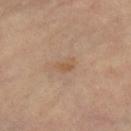{"biopsy_status": "not biopsied; imaged during a skin examination", "patient": {"sex": "female", "age_approx": 65}, "automated_metrics": {"area_mm2_approx": 3.5, "vs_skin_darker_L": 6.0, "vs_skin_contrast_norm": 5.5, "border_irregularity_0_10": 3.0, "color_variation_0_10": 1.5, "nevus_likeness_0_100": 0, "lesion_detection_confidence_0_100": 100}, "image": {"source": "total-body photography crop", "field_of_view_mm": 15}, "lesion_size": {"long_diameter_mm_approx": 2.5}, "site": "right lower leg"}